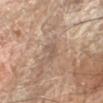Part of a total-body skin-imaging series; this lesion was reviewed on a skin check and was not flagged for biopsy.
Imaged with cross-polarized lighting.
A 15 mm close-up extracted from a 3D total-body photography capture.
A male patient in their mid-60s.
The lesion is on the left forearm.